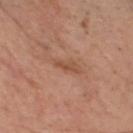notes: catalogued during a skin exam; not biopsied